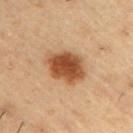The lesion was photographed on a routine skin check and not biopsied; there is no pathology result. About 5 mm across. Automated tile analysis of the lesion measured a footprint of about 14 mm² and a symmetry-axis asymmetry near 0.15. The analysis additionally found a mean CIELAB color near L≈50 a*≈23 b*≈36 and about 16 CIELAB-L* units darker than the surrounding skin. It also reported a border-irregularity index near 2/10 and peripheral color asymmetry of about 1.5. Captured under cross-polarized illumination. A 15 mm close-up tile from a total-body photography series done for melanoma screening. A male patient, aged around 55. From the right upper arm.